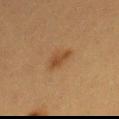follow-up = imaged on a skin check; not biopsied | subject = female, aged around 40 | lesion size = ≈3.5 mm | site = the leg | imaging modality = 15 mm crop, total-body photography | illumination = cross-polarized illumination.